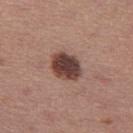biopsy status = total-body-photography surveillance lesion; no biopsy | subject = female, in their mid- to late 30s | body site = the right thigh | lesion size = about 4 mm | acquisition = total-body-photography crop, ~15 mm field of view | illumination = white-light.